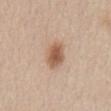Clinical impression: Captured during whole-body skin photography for melanoma surveillance; the lesion was not biopsied. Acquisition and patient details: A region of skin cropped from a whole-body photographic capture, roughly 15 mm wide. The lesion-visualizer software estimated an area of roughly 6.5 mm², an outline eccentricity of about 0.75 (0 = round, 1 = elongated), and a symmetry-axis asymmetry near 0.2. It also reported a border-irregularity index near 1.5/10, a color-variation rating of about 3/10, and a peripheral color-asymmetry measure near 0.5. The lesion's longest dimension is about 3.5 mm. The lesion is located on the abdomen. The tile uses white-light illumination. A male patient, in their 60s.